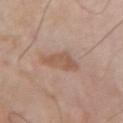The lesion was tiled from a total-body skin photograph and was not biopsied.
The lesion's longest dimension is about 4 mm.
Captured under white-light illumination.
From the arm.
A male patient aged 78 to 82.
The lesion-visualizer software estimated a footprint of about 7.5 mm², an outline eccentricity of about 0.8 (0 = round, 1 = elongated), and a shape-asymmetry score of about 0.35 (0 = symmetric). The software also gave an average lesion color of about L≈55 a*≈18 b*≈28 (CIELAB), about 8 CIELAB-L* units darker than the surrounding skin, and a normalized lesion–skin contrast near 6.5. And it measured a border-irregularity index near 4/10 and internal color variation of about 2.5 on a 0–10 scale.
A lesion tile, about 15 mm wide, cut from a 3D total-body photograph.